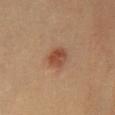Part of a total-body skin-imaging series; this lesion was reviewed on a skin check and was not flagged for biopsy. This is a cross-polarized tile. Longest diameter approximately 2.5 mm. A female subject, aged around 40. A roughly 15 mm field-of-view crop from a total-body skin photograph. Located on the chest.